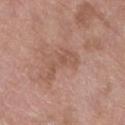{
  "biopsy_status": "not biopsied; imaged during a skin examination",
  "image": {
    "source": "total-body photography crop",
    "field_of_view_mm": 15
  },
  "site": "left lower leg",
  "automated_metrics": {
    "vs_skin_darker_L": 7.0,
    "vs_skin_contrast_norm": 5.0
  },
  "patient": {
    "sex": "female",
    "age_approx": 70
  }
}